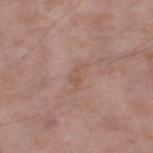Impression:
No biopsy was performed on this lesion — it was imaged during a full skin examination and was not determined to be concerning.
Acquisition and patient details:
The subject is a male aged 53–57. A close-up tile cropped from a whole-body skin photograph, about 15 mm across. The lesion is located on the leg. An algorithmic analysis of the crop reported an area of roughly 2.5 mm². And it measured a color-variation rating of about 0/10.